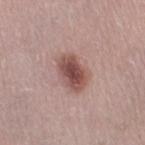Part of a total-body skin-imaging series; this lesion was reviewed on a skin check and was not flagged for biopsy. A female patient about 40 years old. Located on the right thigh. Cropped from a whole-body photographic skin survey; the tile spans about 15 mm. Approximately 4 mm at its widest. This is a white-light tile.